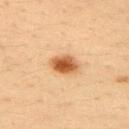This lesion was catalogued during total-body skin photography and was not selected for biopsy. The lesion's longest dimension is about 3.5 mm. Imaged with cross-polarized lighting. This image is a 15 mm lesion crop taken from a total-body photograph. A male subject, aged 33–37. Automated image analysis of the tile measured a normalized lesion–skin contrast near 11. The software also gave lesion-presence confidence of about 100/100. On the upper back.